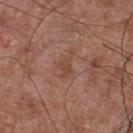Captured during whole-body skin photography for melanoma surveillance; the lesion was not biopsied.
The lesion is located on the chest.
A roughly 15 mm field-of-view crop from a total-body skin photograph.
A male subject in their mid-50s.
This is a white-light tile.